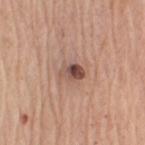Q: Was this lesion biopsied?
A: no biopsy performed (imaged during a skin exam)
Q: Lesion location?
A: the right upper arm
Q: What lighting was used for the tile?
A: white-light
Q: What is the imaging modality?
A: ~15 mm crop, total-body skin-cancer survey
Q: Patient demographics?
A: male, roughly 65 years of age